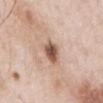Impression: Part of a total-body skin-imaging series; this lesion was reviewed on a skin check and was not flagged for biopsy. Image and clinical context: A region of skin cropped from a whole-body photographic capture, roughly 15 mm wide. The lesion is on the chest. The tile uses white-light illumination. The lesion-visualizer software estimated a lesion area of about 6 mm² and a symmetry-axis asymmetry near 0.2. It also reported a lesion color around L≈55 a*≈19 b*≈28 in CIELAB, about 16 CIELAB-L* units darker than the surrounding skin, and a normalized lesion–skin contrast near 10. The subject is a male approximately 55 years of age. The recorded lesion diameter is about 3.5 mm.